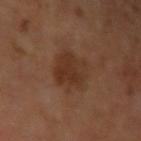  biopsy_status: not biopsied; imaged during a skin examination
  image:
    source: total-body photography crop
    field_of_view_mm: 15
  site: left upper arm
  lesion_size:
    long_diameter_mm_approx: 4.0
  lighting: cross-polarized
  patient:
    sex: male
    age_approx: 65